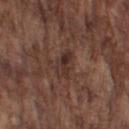Part of a total-body skin-imaging series; this lesion was reviewed on a skin check and was not flagged for biopsy.
Located on the left upper arm.
A 15 mm close-up extracted from a 3D total-body photography capture.
This is a white-light tile.
The lesion-visualizer software estimated a footprint of about 6 mm², a shape eccentricity near 0.8, and two-axis asymmetry of about 0.25. The analysis additionally found internal color variation of about 6 on a 0–10 scale and radial color variation of about 2.5. It also reported a classifier nevus-likeness of about 25/100.
Measured at roughly 3.5 mm in maximum diameter.
A male subject, aged around 75.